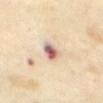workup: no biopsy performed (imaged during a skin exam) | illumination: cross-polarized illumination | lesion size: ~3 mm (longest diameter) | image-analysis metrics: an area of roughly 5 mm² and a symmetry-axis asymmetry near 0.2; a border-irregularity index near 2/10 and a peripheral color-asymmetry measure near 3; a classifier nevus-likeness of about 40/100 | patient: female, in their mid- to late 50s | site: the front of the torso | acquisition: ~15 mm crop, total-body skin-cancer survey.